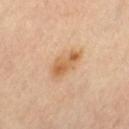Impression:
Part of a total-body skin-imaging series; this lesion was reviewed on a skin check and was not flagged for biopsy.
Background:
This image is a 15 mm lesion crop taken from a total-body photograph. A female patient, aged approximately 65. Approximately 4.5 mm at its widest. From the leg. The tile uses cross-polarized illumination.